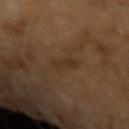{"biopsy_status": "not biopsied; imaged during a skin examination", "lesion_size": {"long_diameter_mm_approx": 3.0}, "lighting": "cross-polarized", "patient": {"sex": "male", "age_approx": 65}, "image": {"source": "total-body photography crop", "field_of_view_mm": 15}}